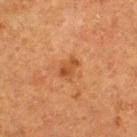Located on the left thigh.
A female patient aged 48–52.
This image is a 15 mm lesion crop taken from a total-body photograph.